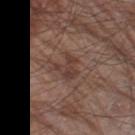biopsy_status: not biopsied; imaged during a skin examination
image:
  source: total-body photography crop
  field_of_view_mm: 15
patient:
  sex: male
  age_approx: 80
site: left thigh
lighting: white-light
lesion_size:
  long_diameter_mm_approx: 3.5